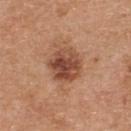| feature | finding |
|---|---|
| follow-up | imaged on a skin check; not biopsied |